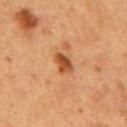{
  "patient": {
    "sex": "male",
    "age_approx": 55
  },
  "lesion_size": {
    "long_diameter_mm_approx": 3.0
  },
  "site": "back",
  "automated_metrics": {
    "border_irregularity_0_10": 2.0,
    "color_variation_0_10": 4.5,
    "nevus_likeness_0_100": 85,
    "lesion_detection_confidence_0_100": 100
  },
  "image": {
    "source": "total-body photography crop",
    "field_of_view_mm": 15
  }
}